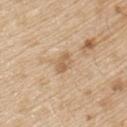  biopsy_status: not biopsied; imaged during a skin examination
  lesion_size:
    long_diameter_mm_approx: 2.5
  lighting: white-light
  patient:
    sex: male
    age_approx: 70
  site: arm
  image:
    source: total-body photography crop
    field_of_view_mm: 15
  automated_metrics:
    eccentricity: 0.75
    shape_asymmetry: 0.2